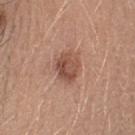This lesion was catalogued during total-body skin photography and was not selected for biopsy.
About 3.5 mm across.
The patient is a female aged 33 to 37.
The lesion is located on the head or neck.
A lesion tile, about 15 mm wide, cut from a 3D total-body photograph.
Captured under white-light illumination.